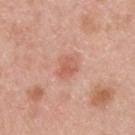{"biopsy_status": "not biopsied; imaged during a skin examination", "patient": {"sex": "male", "age_approx": 45}, "image": {"source": "total-body photography crop", "field_of_view_mm": 15}, "automated_metrics": {"area_mm2_approx": 4.0, "shape_asymmetry": 0.3, "cielab_L": 59, "cielab_a": 26, "cielab_b": 31, "vs_skin_darker_L": 8.0, "nevus_likeness_0_100": 65, "lesion_detection_confidence_0_100": 100}, "lighting": "white-light", "site": "upper back"}